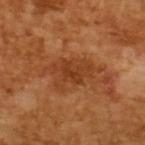Findings:
- image source: total-body-photography crop, ~15 mm field of view
- image-analysis metrics: border irregularity of about 6 on a 0–10 scale, a within-lesion color-variation index near 3.5/10, and peripheral color asymmetry of about 1
- size: ≈5.5 mm
- illumination: cross-polarized
- subject: male, about 65 years old